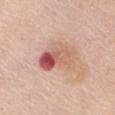{
  "biopsy_status": "not biopsied; imaged during a skin examination",
  "patient": {
    "sex": "female",
    "age_approx": 75
  },
  "lighting": "white-light",
  "site": "abdomen",
  "lesion_size": {
    "long_diameter_mm_approx": 5.0
  },
  "automated_metrics": {
    "border_irregularity_0_10": 2.5,
    "color_variation_0_10": 10.0,
    "peripheral_color_asymmetry": 6.0,
    "nevus_likeness_0_100": 0
  },
  "image": {
    "source": "total-body photography crop",
    "field_of_view_mm": 15
  }
}